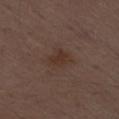Assessment:
Imaged during a routine full-body skin examination; the lesion was not biopsied and no histopathology is available.
Acquisition and patient details:
A male patient, approximately 70 years of age. This image is a 15 mm lesion crop taken from a total-body photograph. Captured under white-light illumination. Approximately 3 mm at its widest. Located on the right thigh.